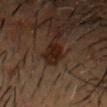Impression:
Imaged during a routine full-body skin examination; the lesion was not biopsied and no histopathology is available.
Background:
A region of skin cropped from a whole-body photographic capture, roughly 15 mm wide. Automated image analysis of the tile measured a lesion area of about 4.5 mm² and a shape-asymmetry score of about 0.3 (0 = symmetric). The software also gave about 7 CIELAB-L* units darker than the surrounding skin and a normalized border contrast of about 10.5. It also reported a nevus-likeness score of about 85/100 and a lesion-detection confidence of about 100/100. Located on the head or neck. Imaged with cross-polarized lighting. The patient is a male aged 48 to 52.